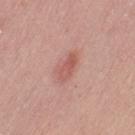The lesion was photographed on a routine skin check and not biopsied; there is no pathology result.
The tile uses white-light illumination.
Located on the left thigh.
A female subject in their 50s.
An algorithmic analysis of the crop reported a lesion area of about 4 mm², an eccentricity of roughly 0.9, and a shape-asymmetry score of about 0.3 (0 = symmetric). The analysis additionally found a border-irregularity index near 3/10, a color-variation rating of about 2.5/10, and peripheral color asymmetry of about 1. It also reported an automated nevus-likeness rating near 70 out of 100 and lesion-presence confidence of about 100/100.
The lesion's longest dimension is about 3.5 mm.
A roughly 15 mm field-of-view crop from a total-body skin photograph.